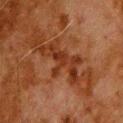• workup — catalogued during a skin exam; not biopsied
• anatomic site — the upper back
• illumination — cross-polarized illumination
• automated lesion analysis — roughly 8 lightness units darker than nearby skin and a normalized border contrast of about 8; a border-irregularity rating of about 10/10 and peripheral color asymmetry of about 0.5
• subject — male, aged 78 to 82
• lesion size — about 6.5 mm
• acquisition — ~15 mm crop, total-body skin-cancer survey